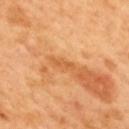A male patient aged approximately 50.
The lesion is on the mid back.
Cropped from a whole-body photographic skin survey; the tile spans about 15 mm.